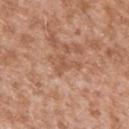Approximately 3 mm at its widest.
Automated tile analysis of the lesion measured an area of roughly 4 mm², an outline eccentricity of about 0.8 (0 = round, 1 = elongated), and a shape-asymmetry score of about 0.65 (0 = symmetric). And it measured a lesion color around L≈55 a*≈22 b*≈32 in CIELAB and roughly 7 lightness units darker than nearby skin. The software also gave a nevus-likeness score of about 0/100 and lesion-presence confidence of about 100/100.
The patient is a male aged around 45.
Cropped from a whole-body photographic skin survey; the tile spans about 15 mm.
The lesion is on the arm.
This is a white-light tile.